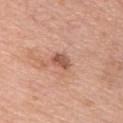biopsy_status: not biopsied; imaged during a skin examination
automated_metrics:
  area_mm2_approx: 3.5
  eccentricity: 0.7
  shape_asymmetry: 0.25
  cielab_L: 54
  cielab_a: 24
  cielab_b: 30
  border_irregularity_0_10: 2.5
  color_variation_0_10: 2.5
  peripheral_color_asymmetry: 1.0
lesion_size:
  long_diameter_mm_approx: 2.5
patient:
  sex: male
  age_approx: 60
image:
  source: total-body photography crop
  field_of_view_mm: 15
site: chest
lighting: white-light